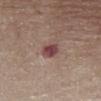This is a white-light tile.
A region of skin cropped from a whole-body photographic capture, roughly 15 mm wide.
The recorded lesion diameter is about 2.5 mm.
The patient is a female approximately 45 years of age.
From the chest.
An algorithmic analysis of the crop reported a shape eccentricity near 0.65 and a shape-asymmetry score of about 0.35 (0 = symmetric). It also reported border irregularity of about 3 on a 0–10 scale, internal color variation of about 3 on a 0–10 scale, and radial color variation of about 1. The analysis additionally found an automated nevus-likeness rating near 15 out of 100 and lesion-presence confidence of about 100/100.